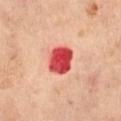This is a cross-polarized tile. About 3.5 mm across. A female patient, aged around 65. A 15 mm close-up extracted from a 3D total-body photography capture. On the abdomen.